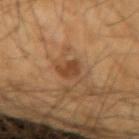<record>
  <biopsy_status>not biopsied; imaged during a skin examination</biopsy_status>
  <lesion_size>
    <long_diameter_mm_approx>3.0</long_diameter_mm_approx>
  </lesion_size>
  <image>
    <source>total-body photography crop</source>
    <field_of_view_mm>15</field_of_view_mm>
  </image>
  <lighting>cross-polarized</lighting>
  <patient>
    <sex>male</sex>
    <age_approx>65</age_approx>
  </patient>
  <site>left forearm</site>
</record>